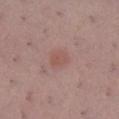Imaged during a routine full-body skin examination; the lesion was not biopsied and no histopathology is available.
Imaged with white-light lighting.
From the leg.
A female patient aged approximately 55.
About 2.5 mm across.
The total-body-photography lesion software estimated a classifier nevus-likeness of about 25/100 and a detector confidence of about 100 out of 100 that the crop contains a lesion.
Cropped from a total-body skin-imaging series; the visible field is about 15 mm.